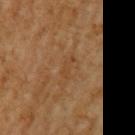Impression: Imaged during a routine full-body skin examination; the lesion was not biopsied and no histopathology is available. Acquisition and patient details: A male subject about 60 years old. Automated tile analysis of the lesion measured an area of roughly 2.5 mm², a shape eccentricity near 0.95, and two-axis asymmetry of about 0.45. Cropped from a total-body skin-imaging series; the visible field is about 15 mm. The lesion is located on the left upper arm. This is a cross-polarized tile.